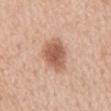Impression: Part of a total-body skin-imaging series; this lesion was reviewed on a skin check and was not flagged for biopsy. Context: Measured at roughly 5 mm in maximum diameter. The patient is a male aged 58–62. Captured under white-light illumination. Located on the mid back. A 15 mm close-up tile from a total-body photography series done for melanoma screening.